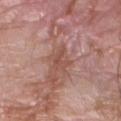{
  "lesion_size": {
    "long_diameter_mm_approx": 3.0
  },
  "image": {
    "source": "total-body photography crop",
    "field_of_view_mm": 15
  },
  "patient": {
    "sex": "male",
    "age_approx": 60
  },
  "lighting": "white-light",
  "site": "right forearm"
}